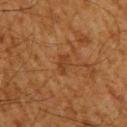Q: Patient demographics?
A: male, about 60 years old
Q: How was this image acquired?
A: ~15 mm tile from a whole-body skin photo
Q: Lesion location?
A: the upper back
Q: What is the lesion's diameter?
A: about 2.5 mm